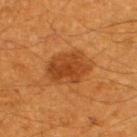Impression:
Captured during whole-body skin photography for melanoma surveillance; the lesion was not biopsied.
Background:
Longest diameter approximately 5 mm. From the upper back. A male patient approximately 60 years of age. A close-up tile cropped from a whole-body skin photograph, about 15 mm across. Captured under cross-polarized illumination.